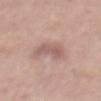Captured during whole-body skin photography for melanoma surveillance; the lesion was not biopsied. Cropped from a total-body skin-imaging series; the visible field is about 15 mm. The lesion is on the abdomen. The subject is a male approximately 50 years of age. Imaged with white-light lighting.